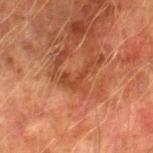Notes:
– notes: no biopsy performed (imaged during a skin exam)